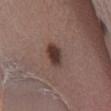biopsy_status: not biopsied; imaged during a skin examination
patient:
  sex: male
  age_approx: 40
image:
  source: total-body photography crop
  field_of_view_mm: 15
site: left lower leg